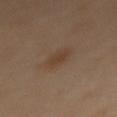Q: Was this lesion biopsied?
A: total-body-photography surveillance lesion; no biopsy
Q: Automated lesion metrics?
A: a shape eccentricity near 0.65; a mean CIELAB color near L≈41 a*≈16 b*≈28, a lesion–skin lightness drop of about 6, and a normalized lesion–skin contrast near 6; peripheral color asymmetry of about 0.5; a classifier nevus-likeness of about 60/100 and a lesion-detection confidence of about 100/100
Q: What kind of image is this?
A: ~15 mm tile from a whole-body skin photo
Q: How large is the lesion?
A: about 3 mm
Q: What are the patient's age and sex?
A: female, approximately 55 years of age
Q: What is the anatomic site?
A: the abdomen
Q: What lighting was used for the tile?
A: cross-polarized